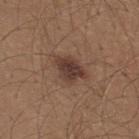Findings:
– follow-up · imaged on a skin check; not biopsied